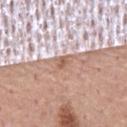Impression: Recorded during total-body skin imaging; not selected for excision or biopsy. Background: A male subject, about 55 years old. This is a white-light tile. Measured at roughly 2.5 mm in maximum diameter. Cropped from a total-body skin-imaging series; the visible field is about 15 mm. Automated image analysis of the tile measured a lesion area of about 3.5 mm². It also reported a classifier nevus-likeness of about 0/100 and a lesion-detection confidence of about 90/100. Located on the chest.